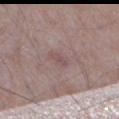Notes:
- follow-up — catalogued during a skin exam; not biopsied
- body site — the left thigh
- image source — 15 mm crop, total-body photography
- lesion size — about 3 mm
- patient — male, aged approximately 65